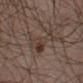biopsy status: no biopsy performed (imaged during a skin exam); image source: ~15 mm crop, total-body skin-cancer survey; anatomic site: the leg; lighting: cross-polarized illumination; subject: male, aged approximately 40; lesion size: about 5.5 mm.